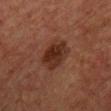The lesion was photographed on a routine skin check and not biopsied; there is no pathology result. From the chest. Imaged with cross-polarized lighting. Cropped from a total-body skin-imaging series; the visible field is about 15 mm. A female patient about 70 years old. Approximately 4.5 mm at its widest.